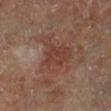Recorded during total-body skin imaging; not selected for excision or biopsy. A 15 mm close-up extracted from a 3D total-body photography capture. The lesion is on the leg. The subject is a male about 70 years old.